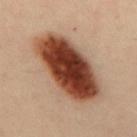Assessment: Captured during whole-body skin photography for melanoma surveillance; the lesion was not biopsied. Acquisition and patient details: A male patient about 50 years old. The lesion is located on the back. Captured under cross-polarized illumination. Measured at roughly 9.5 mm in maximum diameter. Cropped from a whole-body photographic skin survey; the tile spans about 15 mm.